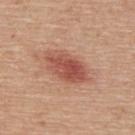Clinical impression: Captured during whole-body skin photography for melanoma surveillance; the lesion was not biopsied. Context: Imaged with white-light lighting. An algorithmic analysis of the crop reported a lesion area of about 13 mm² and a symmetry-axis asymmetry near 0.15. The analysis additionally found a lesion color around L≈53 a*≈27 b*≈29 in CIELAB and a lesion–skin lightness drop of about 12. It also reported a border-irregularity rating of about 2/10 and internal color variation of about 4.5 on a 0–10 scale. On the back. The patient is a male approximately 55 years of age. A 15 mm crop from a total-body photograph taken for skin-cancer surveillance.